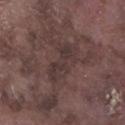notes = total-body-photography surveillance lesion; no biopsy
anatomic site = the left lower leg
subject = male, aged around 75
lesion diameter = ~5 mm (longest diameter)
illumination = white-light
imaging modality = ~15 mm crop, total-body skin-cancer survey